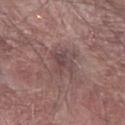patient: male, aged 78 to 82 | image source: total-body-photography crop, ~15 mm field of view | anatomic site: the right forearm.